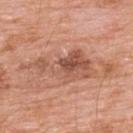| field | value |
|---|---|
| workup | imaged on a skin check; not biopsied |
| body site | the upper back |
| image | ~15 mm tile from a whole-body skin photo |
| patient | male, about 60 years old |